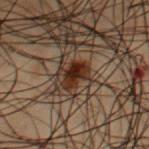Captured during whole-body skin photography for melanoma surveillance; the lesion was not biopsied. From the chest. About 3 mm across. A lesion tile, about 15 mm wide, cut from a 3D total-body photograph. A male patient approximately 55 years of age. Imaged with cross-polarized lighting.